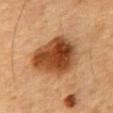follow-up: catalogued during a skin exam; not biopsied | diameter: ≈7 mm | lighting: cross-polarized illumination | automated lesion analysis: an area of roughly 26 mm², a shape eccentricity near 0.7, and a symmetry-axis asymmetry near 0.2 | subject: male, aged 83 or older | anatomic site: the chest | imaging modality: ~15 mm crop, total-body skin-cancer survey.